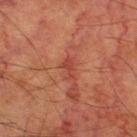Imaged during a routine full-body skin examination; the lesion was not biopsied and no histopathology is available. This is a cross-polarized tile. The subject is a male in their 80s. A 15 mm close-up tile from a total-body photography series done for melanoma screening. On the right thigh. An algorithmic analysis of the crop reported a footprint of about 3.5 mm², an eccentricity of roughly 0.85, and a shape-asymmetry score of about 0.45 (0 = symmetric). The analysis additionally found an average lesion color of about L≈35 a*≈26 b*≈26 (CIELAB) and a lesion–skin lightness drop of about 6. The software also gave a border-irregularity index near 4.5/10 and a within-lesion color-variation index near 0.5/10. The software also gave an automated nevus-likeness rating near 0 out of 100 and a lesion-detection confidence of about 100/100.